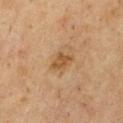Assessment: Imaged during a routine full-body skin examination; the lesion was not biopsied and no histopathology is available. Image and clinical context: This is a cross-polarized tile. The total-body-photography lesion software estimated a lesion area of about 4 mm², an outline eccentricity of about 0.75 (0 = round, 1 = elongated), and a symmetry-axis asymmetry near 0.4. The software also gave a border-irregularity rating of about 3.5/10 and peripheral color asymmetry of about 0.5. And it measured a classifier nevus-likeness of about 35/100. Cropped from a whole-body photographic skin survey; the tile spans about 15 mm. On the chest. The patient is a male aged 68–72. The lesion's longest dimension is about 3 mm.